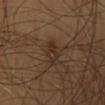Assessment:
The lesion was photographed on a routine skin check and not biopsied; there is no pathology result.
Background:
Automated tile analysis of the lesion measured an eccentricity of roughly 0.85 and a shape-asymmetry score of about 0.15 (0 = symmetric). It also reported a mean CIELAB color near L≈30 a*≈15 b*≈26, roughly 6 lightness units darker than nearby skin, and a lesion-to-skin contrast of about 6.5 (normalized; higher = more distinct). The software also gave a border-irregularity rating of about 2.5/10, internal color variation of about 4.5 on a 0–10 scale, and a peripheral color-asymmetry measure near 2. A close-up tile cropped from a whole-body skin photograph, about 15 mm across. A female subject, aged 38 to 42. Approximately 3.5 mm at its widest. Captured under cross-polarized illumination. The lesion is on the chest.